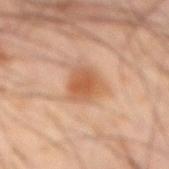body site: the abdomen | patient: male, aged 63 to 67 | automated metrics: internal color variation of about 3 on a 0–10 scale and peripheral color asymmetry of about 1; an automated nevus-likeness rating near 90 out of 100 and a detector confidence of about 100 out of 100 that the crop contains a lesion | image: ~15 mm crop, total-body skin-cancer survey | lighting: cross-polarized illumination | lesion size: ≈3.5 mm.